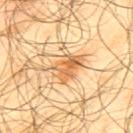follow-up: total-body-photography surveillance lesion; no biopsy
patient: male, aged 63–67
image source: ~15 mm crop, total-body skin-cancer survey
body site: the upper back